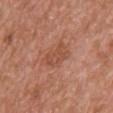No biopsy was performed on this lesion — it was imaged during a full skin examination and was not determined to be concerning. On the upper back. The subject is a male in their mid- to late 70s. Automated image analysis of the tile measured a nevus-likeness score of about 0/100 and a detector confidence of about 100 out of 100 that the crop contains a lesion. Cropped from a whole-body photographic skin survey; the tile spans about 15 mm. This is a white-light tile.